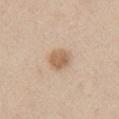Imaged during a routine full-body skin examination; the lesion was not biopsied and no histopathology is available. This image is a 15 mm lesion crop taken from a total-body photograph. Longest diameter approximately 3 mm. The lesion is located on the right upper arm. A female patient approximately 45 years of age. Automated tile analysis of the lesion measured a footprint of about 6 mm² and a shape eccentricity near 0.5. And it measured a border-irregularity rating of about 1.5/10. It also reported an automated nevus-likeness rating near 90 out of 100 and lesion-presence confidence of about 100/100. The tile uses white-light illumination.